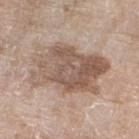Imaged during a routine full-body skin examination; the lesion was not biopsied and no histopathology is available.
A female patient aged 73–77.
The lesion is located on the left lower leg.
A region of skin cropped from a whole-body photographic capture, roughly 15 mm wide.